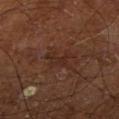biopsy status: no biopsy performed (imaged during a skin exam); lesion size: about 3.5 mm; patient: male, aged 63 to 67; imaging modality: total-body-photography crop, ~15 mm field of view; illumination: cross-polarized illumination; location: the right lower leg.